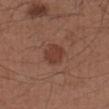Q: Is there a histopathology result?
A: no biopsy performed (imaged during a skin exam)
Q: Lesion location?
A: the left forearm
Q: What is the lesion's diameter?
A: ~3 mm (longest diameter)
Q: How was this image acquired?
A: ~15 mm tile from a whole-body skin photo
Q: Illumination type?
A: white-light illumination
Q: Who is the patient?
A: male, approximately 55 years of age